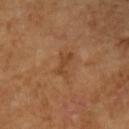Case summary:
- follow-up — no biopsy performed (imaged during a skin exam)
- site — the arm
- tile lighting — cross-polarized
- diameter — ~3.5 mm (longest diameter)
- acquisition — ~15 mm tile from a whole-body skin photo
- subject — female, aged approximately 70